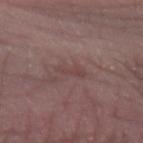Clinical impression: Recorded during total-body skin imaging; not selected for excision or biopsy. Clinical summary: Automated tile analysis of the lesion measured an eccentricity of roughly 0.95 and two-axis asymmetry of about 0.45. The analysis additionally found an average lesion color of about L≈42 a*≈19 b*≈19 (CIELAB) and a lesion-to-skin contrast of about 5 (normalized; higher = more distinct). The software also gave an automated nevus-likeness rating near 0 out of 100. Longest diameter approximately 3 mm. Imaged with white-light lighting. A female patient aged 48 to 52. A region of skin cropped from a whole-body photographic capture, roughly 15 mm wide. From the left forearm.